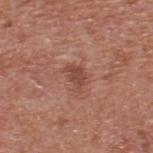The lesion is on the back. A region of skin cropped from a whole-body photographic capture, roughly 15 mm wide. Automated image analysis of the tile measured a footprint of about 4 mm² and a shape eccentricity near 0.75. And it measured a mean CIELAB color near L≈46 a*≈24 b*≈27, roughly 9 lightness units darker than nearby skin, and a normalized lesion–skin contrast near 7. It also reported a color-variation rating of about 1.5/10 and peripheral color asymmetry of about 0.5. The analysis additionally found a nevus-likeness score of about 0/100 and a lesion-detection confidence of about 100/100. Captured under white-light illumination. A male patient in their mid- to late 60s. Approximately 3 mm at its widest.